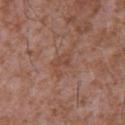No biopsy was performed on this lesion — it was imaged during a full skin examination and was not determined to be concerning. A male patient aged around 45. A 15 mm crop from a total-body photograph taken for skin-cancer surveillance. Located on the chest.